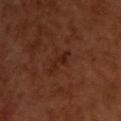Assessment: The lesion was photographed on a routine skin check and not biopsied; there is no pathology result. Background: This is a cross-polarized tile. A male patient, roughly 50 years of age. Measured at roughly 3.5 mm in maximum diameter. From the upper back. An algorithmic analysis of the crop reported a shape eccentricity near 0.9 and a shape-asymmetry score of about 0.4 (0 = symmetric). The analysis additionally found an average lesion color of about L≈24 a*≈22 b*≈27 (CIELAB) and a lesion–skin lightness drop of about 6. It also reported a nevus-likeness score of about 15/100 and lesion-presence confidence of about 100/100. This image is a 15 mm lesion crop taken from a total-body photograph.